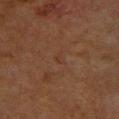Context:
The lesion is on the upper back. The patient is a female about 80 years old. Measured at roughly 1.5 mm in maximum diameter. A lesion tile, about 15 mm wide, cut from a 3D total-body photograph. Imaged with cross-polarized lighting.